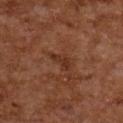Impression:
The lesion was tiled from a total-body skin photograph and was not biopsied.
Image and clinical context:
A female patient, aged 58–62. The lesion is on the upper back. A 15 mm close-up tile from a total-body photography series done for melanoma screening.